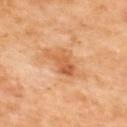Q: Is there a histopathology result?
A: catalogued during a skin exam; not biopsied
Q: Where on the body is the lesion?
A: the upper back
Q: What is the imaging modality?
A: total-body-photography crop, ~15 mm field of view